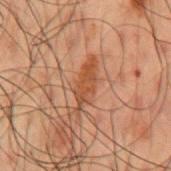Q: What lighting was used for the tile?
A: cross-polarized illumination
Q: What is the imaging modality?
A: ~15 mm tile from a whole-body skin photo
Q: Lesion size?
A: ~5.5 mm (longest diameter)
Q: What is the anatomic site?
A: the mid back
Q: Who is the patient?
A: male, in their 50s